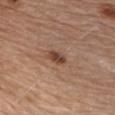• biopsy status · imaged on a skin check; not biopsied
• subject · male, approximately 80 years of age
• automated lesion analysis · a lesion color around L≈44 a*≈21 b*≈29 in CIELAB and a normalized border contrast of about 9; a nevus-likeness score of about 75/100 and a lesion-detection confidence of about 100/100
• diameter · ~3 mm (longest diameter)
• anatomic site · the chest
• imaging modality · total-body-photography crop, ~15 mm field of view
• tile lighting · white-light